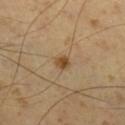Captured during whole-body skin photography for melanoma surveillance; the lesion was not biopsied.
The total-body-photography lesion software estimated an eccentricity of roughly 0.5 and a shape-asymmetry score of about 0.3 (0 = symmetric). The analysis additionally found an average lesion color of about L≈47 a*≈17 b*≈36 (CIELAB), roughly 11 lightness units darker than nearby skin, and a lesion-to-skin contrast of about 9 (normalized; higher = more distinct).
A male subject, approximately 55 years of age.
Imaged with cross-polarized lighting.
Located on the right lower leg.
A 15 mm crop from a total-body photograph taken for skin-cancer surveillance.